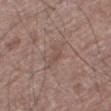Notes:
• follow-up: imaged on a skin check; not biopsied
• subject: male, in their mid- to late 70s
• site: the leg
• imaging modality: ~15 mm crop, total-body skin-cancer survey
• automated lesion analysis: an area of roughly 2 mm², an eccentricity of roughly 0.9, and a shape-asymmetry score of about 0.5 (0 = symmetric); a nevus-likeness score of about 0/100 and a detector confidence of about 95 out of 100 that the crop contains a lesion
• tile lighting: white-light illumination